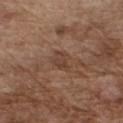workup — imaged on a skin check; not biopsied | size — ~2.5 mm (longest diameter) | subject — male, aged around 75 | anatomic site — the chest | image source — ~15 mm tile from a whole-body skin photo.